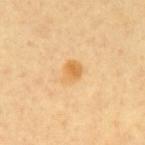A 15 mm close-up tile from a total-body photography series done for melanoma screening. Automated image analysis of the tile measured a lesion area of about 5 mm², an outline eccentricity of about 0.65 (0 = round, 1 = elongated), and two-axis asymmetry of about 0.2. It also reported a border-irregularity rating of about 2/10, a within-lesion color-variation index near 4.5/10, and radial color variation of about 1.5. The lesion's longest dimension is about 3 mm. The subject is about 60 years old. The tile uses cross-polarized illumination. On the mid back.